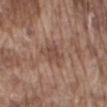The lesion is on the mid back. The total-body-photography lesion software estimated a shape eccentricity near 0.5 and a shape-asymmetry score of about 0.3 (0 = symmetric). The software also gave a nevus-likeness score of about 0/100 and a detector confidence of about 100 out of 100 that the crop contains a lesion. Approximately 2.5 mm at its widest. This is a white-light tile. The patient is a male approximately 75 years of age. A roughly 15 mm field-of-view crop from a total-body skin photograph.